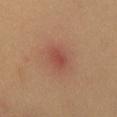Q: Is there a histopathology result?
A: no biopsy performed (imaged during a skin exam)
Q: What is the imaging modality?
A: ~15 mm crop, total-body skin-cancer survey
Q: Where on the body is the lesion?
A: the chest
Q: What did automated image analysis measure?
A: a within-lesion color-variation index near 2/10 and a peripheral color-asymmetry measure near 0.5
Q: How was the tile lit?
A: cross-polarized
Q: What is the lesion's diameter?
A: ~3 mm (longest diameter)
Q: What are the patient's age and sex?
A: male, aged approximately 40